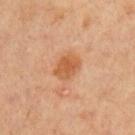  biopsy_status: not biopsied; imaged during a skin examination
  image:
    source: total-body photography crop
    field_of_view_mm: 15
  patient:
    sex: male
    age_approx: 60
  site: left upper arm